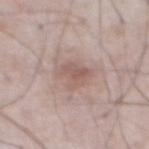Q: Is there a histopathology result?
A: imaged on a skin check; not biopsied
Q: Automated lesion metrics?
A: an average lesion color of about L≈57 a*≈17 b*≈23 (CIELAB), a lesion–skin lightness drop of about 8, and a lesion-to-skin contrast of about 5.5 (normalized; higher = more distinct); a nevus-likeness score of about 30/100
Q: What are the patient's age and sex?
A: male, roughly 75 years of age
Q: Lesion size?
A: about 3.5 mm
Q: What lighting was used for the tile?
A: white-light illumination
Q: How was this image acquired?
A: ~15 mm crop, total-body skin-cancer survey
Q: What is the anatomic site?
A: the abdomen